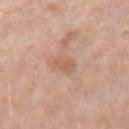Notes:
* patient: female, aged 38 to 42
* image source: 15 mm crop, total-body photography
* anatomic site: the right forearm